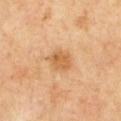Recorded during total-body skin imaging; not selected for excision or biopsy.